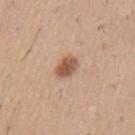The lesion was photographed on a routine skin check and not biopsied; there is no pathology result. Imaged with white-light lighting. A male subject roughly 60 years of age. A close-up tile cropped from a whole-body skin photograph, about 15 mm across. The lesion's longest dimension is about 3 mm. The lesion is on the left upper arm.